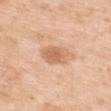Clinical impression: The lesion was tiled from a total-body skin photograph and was not biopsied. Context: The lesion is on the upper back. A region of skin cropped from a whole-body photographic capture, roughly 15 mm wide. Imaged with white-light lighting. A female subject aged approximately 40.